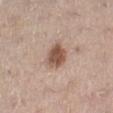Clinical impression: Captured during whole-body skin photography for melanoma surveillance; the lesion was not biopsied. Context: A female subject, aged 38 to 42. A region of skin cropped from a whole-body photographic capture, roughly 15 mm wide. Longest diameter approximately 3 mm. The lesion is located on the left lower leg. Imaged with white-light lighting.